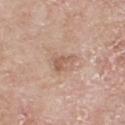Q: Was a biopsy performed?
A: no biopsy performed (imaged during a skin exam)
Q: Where on the body is the lesion?
A: the back
Q: What is the imaging modality?
A: total-body-photography crop, ~15 mm field of view
Q: What is the lesion's diameter?
A: about 3 mm
Q: Who is the patient?
A: male, about 65 years old
Q: How was the tile lit?
A: white-light